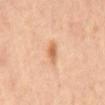The lesion was photographed on a routine skin check and not biopsied; there is no pathology result. Measured at roughly 3 mm in maximum diameter. A 15 mm close-up tile from a total-body photography series done for melanoma screening. Captured under cross-polarized illumination. A male subject, aged approximately 60. Located on the mid back. The lesion-visualizer software estimated a footprint of about 3.5 mm² and an eccentricity of roughly 0.85. The software also gave a mean CIELAB color near L≈62 a*≈23 b*≈37, about 11 CIELAB-L* units darker than the surrounding skin, and a lesion-to-skin contrast of about 7.5 (normalized; higher = more distinct). The software also gave a border-irregularity index near 3/10, a within-lesion color-variation index near 1.5/10, and peripheral color asymmetry of about 0.5.